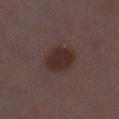Clinical impression:
The lesion was photographed on a routine skin check and not biopsied; there is no pathology result.
Acquisition and patient details:
Cropped from a whole-body photographic skin survey; the tile spans about 15 mm. On the right thigh. The subject is a female about 30 years old.